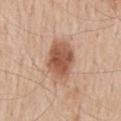- follow-up: no biopsy performed (imaged during a skin exam)
- location: the mid back
- patient: male, in their 60s
- automated lesion analysis: a lesion area of about 15 mm² and a shape-asymmetry score of about 0.15 (0 = symmetric)
- tile lighting: white-light
- image: total-body-photography crop, ~15 mm field of view
- lesion diameter: ≈5 mm